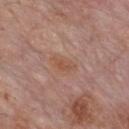* follow-up: total-body-photography surveillance lesion; no biopsy
* automated metrics: an outline eccentricity of about 0.8 (0 = round, 1 = elongated) and a shape-asymmetry score of about 0.3 (0 = symmetric); a mean CIELAB color near L≈52 a*≈21 b*≈29, about 6 CIELAB-L* units darker than the surrounding skin, and a normalized border contrast of about 6; a border-irregularity rating of about 2.5/10, a color-variation rating of about 2.5/10, and peripheral color asymmetry of about 1; an automated nevus-likeness rating near 0 out of 100 and lesion-presence confidence of about 100/100
* subject: male, aged 58–62
* site: the chest
* diameter: ≈3 mm
* image: ~15 mm crop, total-body skin-cancer survey